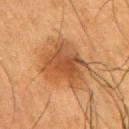Context:
A male patient, aged 48–52. The tile uses cross-polarized illumination. The lesion-visualizer software estimated a symmetry-axis asymmetry near 0.3. And it measured an average lesion color of about L≈41 a*≈20 b*≈32 (CIELAB), a lesion–skin lightness drop of about 9, and a normalized lesion–skin contrast near 7.5. The lesion is on the right upper arm. Measured at roughly 6.5 mm in maximum diameter. A 15 mm close-up tile from a total-body photography series done for melanoma screening.